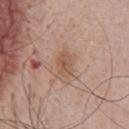{
  "biopsy_status": "not biopsied; imaged during a skin examination",
  "site": "chest",
  "patient": {
    "sex": "male",
    "age_approx": 60
  },
  "image": {
    "source": "total-body photography crop",
    "field_of_view_mm": 15
  }
}